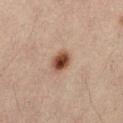Acquisition and patient details: The lesion's longest dimension is about 2.5 mm. The subject is a male about 30 years old. Cropped from a whole-body photographic skin survey; the tile spans about 15 mm. Automated tile analysis of the lesion measured an area of roughly 5 mm² and two-axis asymmetry of about 0.15. And it measured a mean CIELAB color near L≈38 a*≈19 b*≈25 and about 15 CIELAB-L* units darker than the surrounding skin. The lesion is located on the left thigh.